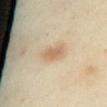Q: Is there a histopathology result?
A: total-body-photography surveillance lesion; no biopsy
Q: What is the anatomic site?
A: the chest
Q: What did automated image analysis measure?
A: a shape eccentricity near 0.7; an average lesion color of about L≈66 a*≈16 b*≈34 (CIELAB); a lesion-detection confidence of about 100/100
Q: Patient demographics?
A: female, aged approximately 55
Q: What lighting was used for the tile?
A: cross-polarized
Q: What kind of image is this?
A: total-body-photography crop, ~15 mm field of view